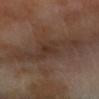<case>
  <biopsy_status>not biopsied; imaged during a skin examination</biopsy_status>
  <patient>
    <sex>male</sex>
    <age_approx>55</age_approx>
  </patient>
  <lesion_size>
    <long_diameter_mm_approx>8.5</long_diameter_mm_approx>
  </lesion_size>
  <site>right forearm</site>
  <image>
    <source>total-body photography crop</source>
    <field_of_view_mm>15</field_of_view_mm>
  </image>
  <lighting>cross-polarized</lighting>
</case>